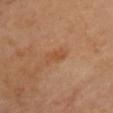biopsy status = imaged on a skin check; not biopsied | image source = 15 mm crop, total-body photography | anatomic site = the chest | TBP lesion metrics = a nevus-likeness score of about 5/100 and lesion-presence confidence of about 100/100 | patient = female, aged around 60 | lighting = cross-polarized.